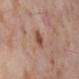notes = catalogued during a skin exam; not biopsied | location = the leg | lesion diameter = about 2.5 mm | image = ~15 mm crop, total-body skin-cancer survey | subject = female, aged 53–57 | image-analysis metrics = an area of roughly 3.5 mm² and an eccentricity of roughly 0.65; a border-irregularity rating of about 2/10, a within-lesion color-variation index near 2.5/10, and a peripheral color-asymmetry measure near 1 | lighting = cross-polarized.